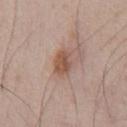Notes:
- follow-up: total-body-photography surveillance lesion; no biopsy
- acquisition: ~15 mm crop, total-body skin-cancer survey
- lesion diameter: ~2.5 mm (longest diameter)
- lighting: white-light
- subject: male, aged around 50
- image-analysis metrics: a lesion area of about 5 mm² and a symmetry-axis asymmetry near 0.2; a normalized lesion–skin contrast near 8; a border-irregularity index near 2/10, internal color variation of about 3 on a 0–10 scale, and radial color variation of about 1; a detector confidence of about 100 out of 100 that the crop contains a lesion
- site: the front of the torso